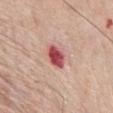follow-up — catalogued during a skin exam; not biopsied | image — ~15 mm crop, total-body skin-cancer survey | lesion size — ≈3.5 mm | lighting — white-light illumination | site — the chest | patient — male, about 80 years old.